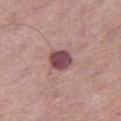follow-up — imaged on a skin check; not biopsied | site — the leg | patient — male, roughly 75 years of age | imaging modality — total-body-photography crop, ~15 mm field of view.